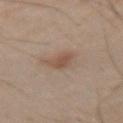Imaged during a routine full-body skin examination; the lesion was not biopsied and no histopathology is available.
Located on the left upper arm.
Automated tile analysis of the lesion measured a lesion area of about 4.5 mm² and two-axis asymmetry of about 0.25. The software also gave a lesion color around L≈40 a*≈14 b*≈23 in CIELAB, roughly 7 lightness units darker than nearby skin, and a normalized border contrast of about 6.5. The software also gave an automated nevus-likeness rating near 70 out of 100 and a lesion-detection confidence of about 100/100.
Cropped from a whole-body photographic skin survey; the tile spans about 15 mm.
The subject is a male approximately 50 years of age.
The recorded lesion diameter is about 3.5 mm.
Captured under cross-polarized illumination.